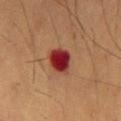Background: A male subject about 55 years old. The tile uses cross-polarized illumination. Longest diameter approximately 3.5 mm. On the abdomen. A lesion tile, about 15 mm wide, cut from a 3D total-body photograph. Automated tile analysis of the lesion measured a lesion color around L≈30 a*≈32 b*≈26 in CIELAB, roughly 16 lightness units darker than nearby skin, and a normalized lesion–skin contrast near 14.